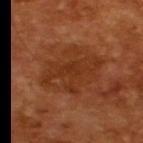<lesion>
<biopsy_status>not biopsied; imaged during a skin examination</biopsy_status>
<patient>
  <sex>male</sex>
  <age_approx>65</age_approx>
</patient>
<lesion_size>
  <long_diameter_mm_approx>7.5</long_diameter_mm_approx>
</lesion_size>
<lighting>cross-polarized</lighting>
<image>
  <source>total-body photography crop</source>
  <field_of_view_mm>15</field_of_view_mm>
</image>
</lesion>